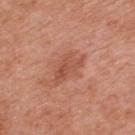Part of a total-body skin-imaging series; this lesion was reviewed on a skin check and was not flagged for biopsy. A roughly 15 mm field-of-view crop from a total-body skin photograph. The total-body-photography lesion software estimated a footprint of about 8 mm², an outline eccentricity of about 0.85 (0 = round, 1 = elongated), and a shape-asymmetry score of about 0.35 (0 = symmetric). And it measured a mean CIELAB color near L≈53 a*≈26 b*≈31 and a lesion–skin lightness drop of about 8. And it measured a color-variation rating of about 4/10 and radial color variation of about 1.5. Approximately 4.5 mm at its widest. The lesion is on the upper back. The tile uses white-light illumination. A male subject aged 68 to 72.